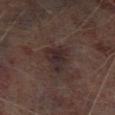Captured during whole-body skin photography for melanoma surveillance; the lesion was not biopsied.
A lesion tile, about 15 mm wide, cut from a 3D total-body photograph.
The tile uses cross-polarized illumination.
A male subject, in their mid-60s.
The lesion is located on the left lower leg.
About 4 mm across.